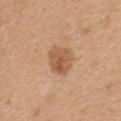This lesion was catalogued during total-body skin photography and was not selected for biopsy. Cropped from a total-body skin-imaging series; the visible field is about 15 mm. A female subject, aged approximately 30. The lesion is on the upper back.